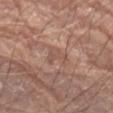workup = total-body-photography surveillance lesion; no biopsy
lesion diameter = ≈3 mm
acquisition = 15 mm crop, total-body photography
tile lighting = white-light
patient = male, aged approximately 80
location = the left forearm